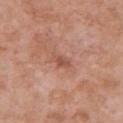Notes:
• biopsy status — total-body-photography surveillance lesion; no biopsy
• acquisition — 15 mm crop, total-body photography
• automated lesion analysis — a lesion-detection confidence of about 100/100
• anatomic site — the chest
• illumination — white-light
• patient — female, about 50 years old